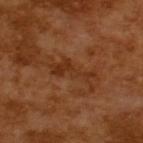Notes:
* notes — catalogued during a skin exam; not biopsied
* patient — male, approximately 65 years of age
* acquisition — ~15 mm tile from a whole-body skin photo
* lesion diameter — ≈6 mm